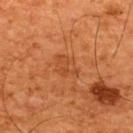Acquisition and patient details: A close-up tile cropped from a whole-body skin photograph, about 15 mm across. From the upper back. About 3 mm across. Automated image analysis of the tile measured a lesion color around L≈39 a*≈24 b*≈35 in CIELAB, about 5 CIELAB-L* units darker than the surrounding skin, and a lesion-to-skin contrast of about 4.5 (normalized; higher = more distinct). A male subject, about 65 years old. Imaged with cross-polarized lighting.